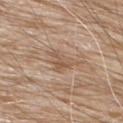Q: Is there a histopathology result?
A: imaged on a skin check; not biopsied
Q: How was this image acquired?
A: total-body-photography crop, ~15 mm field of view
Q: How was the tile lit?
A: white-light illumination
Q: Where on the body is the lesion?
A: the right upper arm
Q: Who is the patient?
A: male, in their 80s
Q: Lesion size?
A: ~3.5 mm (longest diameter)
Q: What did automated image analysis measure?
A: a lesion–skin lightness drop of about 8 and a lesion-to-skin contrast of about 5.5 (normalized; higher = more distinct); a classifier nevus-likeness of about 0/100 and lesion-presence confidence of about 100/100